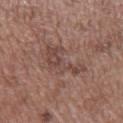Part of a total-body skin-imaging series; this lesion was reviewed on a skin check and was not flagged for biopsy. The lesion is located on the mid back. A male patient approximately 50 years of age. A lesion tile, about 15 mm wide, cut from a 3D total-body photograph. This is a white-light tile.